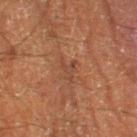{
  "biopsy_status": "not biopsied; imaged during a skin examination",
  "image": {
    "source": "total-body photography crop",
    "field_of_view_mm": 15
  },
  "site": "left lower leg",
  "lighting": "cross-polarized",
  "lesion_size": {
    "long_diameter_mm_approx": 3.0
  },
  "automated_metrics": {
    "cielab_L": 36,
    "cielab_a": 19,
    "cielab_b": 26,
    "vs_skin_darker_L": 5.0,
    "vs_skin_contrast_norm": 5.0,
    "peripheral_color_asymmetry": 0.5,
    "lesion_detection_confidence_0_100": 100
  },
  "patient": {
    "sex": "male",
    "age_approx": 65
  }
}